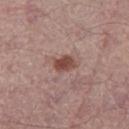Part of a total-body skin-imaging series; this lesion was reviewed on a skin check and was not flagged for biopsy. The lesion-visualizer software estimated a mean CIELAB color near L≈47 a*≈21 b*≈23 and about 12 CIELAB-L* units darker than the surrounding skin. The analysis additionally found a classifier nevus-likeness of about 90/100 and a lesion-detection confidence of about 100/100. A male subject about 55 years old. The lesion is on the leg. A roughly 15 mm field-of-view crop from a total-body skin photograph. About 3 mm across. The tile uses white-light illumination.